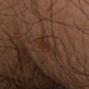Q: Was this lesion biopsied?
A: imaged on a skin check; not biopsied
Q: What is the imaging modality?
A: 15 mm crop, total-body photography
Q: What are the patient's age and sex?
A: female, aged approximately 50
Q: Lesion location?
A: the left forearm